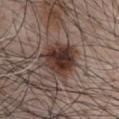Part of a total-body skin-imaging series; this lesion was reviewed on a skin check and was not flagged for biopsy. About 5 mm across. The lesion-visualizer software estimated an outline eccentricity of about 0.35 (0 = round, 1 = elongated) and a shape-asymmetry score of about 0.25 (0 = symmetric). It also reported a within-lesion color-variation index near 7.5/10 and a peripheral color-asymmetry measure near 2.5. On the chest. A 15 mm crop from a total-body photograph taken for skin-cancer surveillance. A male subject, aged approximately 65.